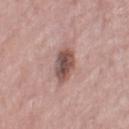- biopsy status · total-body-photography surveillance lesion; no biopsy
- patient · female, aged around 65
- image · 15 mm crop, total-body photography
- site · the right thigh
- lesion size · about 3.5 mm